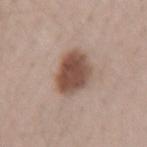biopsy_status: not biopsied; imaged during a skin examination
image:
  source: total-body photography crop
  field_of_view_mm: 15
patient:
  sex: female
  age_approx: 40
site: right forearm
lesion_size:
  long_diameter_mm_approx: 4.5